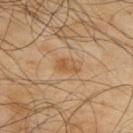Measured at roughly 3 mm in maximum diameter.
On the back.
A male subject, aged 63–67.
A close-up tile cropped from a whole-body skin photograph, about 15 mm across.